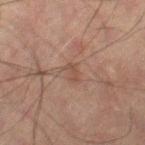Clinical impression:
The lesion was photographed on a routine skin check and not biopsied; there is no pathology result.
Background:
The lesion is on the left leg. Imaged with cross-polarized lighting. A 15 mm crop from a total-body photograph taken for skin-cancer surveillance. A male patient, aged around 60. The lesion-visualizer software estimated an automated nevus-likeness rating near 0 out of 100 and lesion-presence confidence of about 100/100. The recorded lesion diameter is about 2.5 mm.